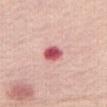This lesion was catalogued during total-body skin photography and was not selected for biopsy. A 15 mm crop from a total-body photograph taken for skin-cancer surveillance. The lesion-visualizer software estimated a lesion area of about 5 mm² and a shape eccentricity near 0.7. The analysis additionally found a nevus-likeness score of about 0/100 and a detector confidence of about 100 out of 100 that the crop contains a lesion. A female subject aged 48 to 52. Located on the abdomen.